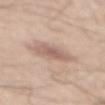Impression:
The lesion was photographed on a routine skin check and not biopsied; there is no pathology result.